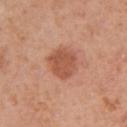<tbp_lesion>
  <lesion_size>
    <long_diameter_mm_approx>3.5</long_diameter_mm_approx>
  </lesion_size>
  <lighting>white-light</lighting>
  <site>left upper arm</site>
  <patient>
    <sex>female</sex>
    <age_approx>40</age_approx>
  </patient>
  <image>
    <source>total-body photography crop</source>
    <field_of_view_mm>15</field_of_view_mm>
  </image>
</tbp_lesion>